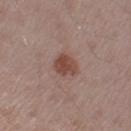Captured during whole-body skin photography for melanoma surveillance; the lesion was not biopsied. This is a white-light tile. From the left upper arm. Longest diameter approximately 3 mm. The subject is a male aged 53 to 57. A region of skin cropped from a whole-body photographic capture, roughly 15 mm wide.